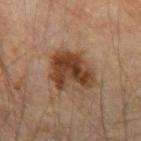Recorded during total-body skin imaging; not selected for excision or biopsy. This is a cross-polarized tile. The lesion is on the right forearm. The lesion's longest dimension is about 5 mm. A male subject, in their mid-40s. The total-body-photography lesion software estimated a lesion color around L≈32 a*≈18 b*≈27 in CIELAB, roughly 13 lightness units darker than nearby skin, and a normalized border contrast of about 11.5. The software also gave a border-irregularity index near 5/10 and a color-variation rating of about 4/10. It also reported a nevus-likeness score of about 90/100 and a detector confidence of about 100 out of 100 that the crop contains a lesion. A close-up tile cropped from a whole-body skin photograph, about 15 mm across.